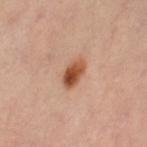Recorded during total-body skin imaging; not selected for excision or biopsy.
A female patient, in their 40s.
The lesion is on the right leg.
A 15 mm close-up extracted from a 3D total-body photography capture.